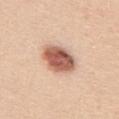Q: Was a biopsy performed?
A: catalogued during a skin exam; not biopsied
Q: What kind of image is this?
A: ~15 mm tile from a whole-body skin photo
Q: How was the tile lit?
A: white-light illumination
Q: How large is the lesion?
A: about 4 mm
Q: What is the anatomic site?
A: the chest
Q: Who is the patient?
A: male, aged approximately 45